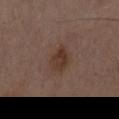Q: Is there a histopathology result?
A: imaged on a skin check; not biopsied